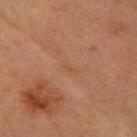Captured during whole-body skin photography for melanoma surveillance; the lesion was not biopsied. A 15 mm close-up tile from a total-body photography series done for melanoma screening. A female subject about 40 years old. Imaged with cross-polarized lighting. From the right forearm.